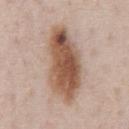<record>
<biopsy_status>not biopsied; imaged during a skin examination</biopsy_status>
<lighting>white-light</lighting>
<site>abdomen</site>
<lesion_size>
  <long_diameter_mm_approx>9.0</long_diameter_mm_approx>
</lesion_size>
<image>
  <source>total-body photography crop</source>
  <field_of_view_mm>15</field_of_view_mm>
</image>
<patient>
  <sex>male</sex>
  <age_approx>60</age_approx>
</patient>
</record>